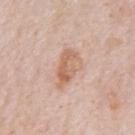biopsy_status: not biopsied; imaged during a skin examination
patient:
  sex: male
  age_approx: 80
image:
  source: total-body photography crop
  field_of_view_mm: 15
site: chest